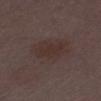Q: Was a biopsy performed?
A: imaged on a skin check; not biopsied
Q: What is the anatomic site?
A: the left thigh
Q: How was this image acquired?
A: ~15 mm crop, total-body skin-cancer survey
Q: What is the lesion's diameter?
A: ~4 mm (longest diameter)
Q: Who is the patient?
A: female, about 30 years old
Q: What did automated image analysis measure?
A: a lesion area of about 8.5 mm² and an eccentricity of roughly 0.8; an average lesion color of about L≈30 a*≈15 b*≈18 (CIELAB) and a lesion-to-skin contrast of about 6 (normalized; higher = more distinct); a border-irregularity rating of about 3/10, internal color variation of about 1.5 on a 0–10 scale, and a peripheral color-asymmetry measure near 0.5